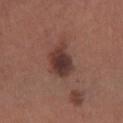Part of a total-body skin-imaging series; this lesion was reviewed on a skin check and was not flagged for biopsy. Located on the right lower leg. The recorded lesion diameter is about 4.5 mm. A female patient aged 53–57. Imaged with white-light lighting. A close-up tile cropped from a whole-body skin photograph, about 15 mm across.